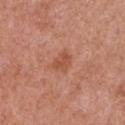Part of a total-body skin-imaging series; this lesion was reviewed on a skin check and was not flagged for biopsy.
Cropped from a whole-body photographic skin survey; the tile spans about 15 mm.
Approximately 2.5 mm at its widest.
The subject is a female approximately 50 years of age.
The lesion is on the chest.
An algorithmic analysis of the crop reported a lesion area of about 4 mm² and a symmetry-axis asymmetry near 0.3. It also reported a lesion color around L≈52 a*≈27 b*≈32 in CIELAB, a lesion–skin lightness drop of about 8, and a normalized border contrast of about 6. The software also gave a border-irregularity index near 2.5/10 and internal color variation of about 1 on a 0–10 scale.